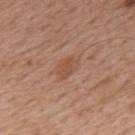size: about 3 mm
subject: male, aged approximately 60
tile lighting: white-light
TBP lesion metrics: a lesion color around L≈51 a*≈22 b*≈30 in CIELAB, a lesion–skin lightness drop of about 7, and a normalized lesion–skin contrast near 5.5
image source: ~15 mm crop, total-body skin-cancer survey
location: the upper back